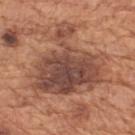Recorded during total-body skin imaging; not selected for excision or biopsy.
The lesion-visualizer software estimated a lesion area of about 42 mm², a shape eccentricity near 0.5, and a shape-asymmetry score of about 0.5 (0 = symmetric). It also reported a mean CIELAB color near L≈47 a*≈22 b*≈28, roughly 12 lightness units darker than nearby skin, and a lesion-to-skin contrast of about 9.5 (normalized; higher = more distinct).
A region of skin cropped from a whole-body photographic capture, roughly 15 mm wide.
A male patient, in their mid- to late 60s.
The lesion is on the mid back.
Longest diameter approximately 9.5 mm.